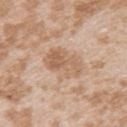Clinical impression: Imaged during a routine full-body skin examination; the lesion was not biopsied and no histopathology is available. Background: The recorded lesion diameter is about 5 mm. A 15 mm close-up extracted from a 3D total-body photography capture. Imaged with white-light lighting. A female patient aged around 25. Located on the left upper arm.